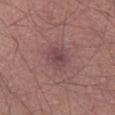workup: total-body-photography surveillance lesion; no biopsy
subject: male, aged approximately 55
automated lesion analysis: a color-variation rating of about 2.5/10
diameter: about 3 mm
illumination: white-light
site: the left thigh
acquisition: total-body-photography crop, ~15 mm field of view